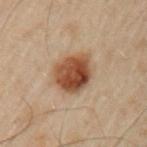Assessment: Recorded during total-body skin imaging; not selected for excision or biopsy. Context: A male patient aged 48 to 52. From the left upper arm. Cropped from a whole-body photographic skin survey; the tile spans about 15 mm. Captured under cross-polarized illumination.